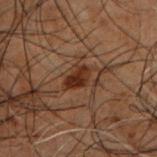Captured during whole-body skin photography for melanoma surveillance; the lesion was not biopsied. The lesion-visualizer software estimated a lesion area of about 4 mm², a shape eccentricity near 0.8, and two-axis asymmetry of about 0.25. The analysis additionally found a lesion color around L≈21 a*≈18 b*≈23 in CIELAB, roughly 9 lightness units darker than nearby skin, and a normalized lesion–skin contrast near 11. And it measured a classifier nevus-likeness of about 80/100 and a detector confidence of about 100 out of 100 that the crop contains a lesion. Captured under cross-polarized illumination. Located on the chest. A 15 mm crop from a total-body photograph taken for skin-cancer surveillance. The recorded lesion diameter is about 3 mm. A male patient, approximately 50 years of age.